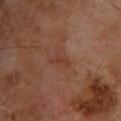follow-up — imaged on a skin check; not biopsied | location — the back | TBP lesion metrics — an area of roughly 2.5 mm² and two-axis asymmetry of about 0.4; a mean CIELAB color near L≈36 a*≈22 b*≈27; a within-lesion color-variation index near 0/10 and radial color variation of about 0; an automated nevus-likeness rating near 0 out of 100 | lesion diameter — ≈3 mm | image — total-body-photography crop, ~15 mm field of view | patient — male, aged approximately 65 | illumination — cross-polarized.